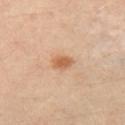A female patient aged around 40. The tile uses cross-polarized illumination. A 15 mm close-up tile from a total-body photography series done for melanoma screening. The lesion is located on the right leg. About 2.5 mm across.